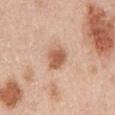| key | value |
|---|---|
| workup | total-body-photography surveillance lesion; no biopsy |
| tile lighting | white-light |
| image | 15 mm crop, total-body photography |
| lesion size | ~3 mm (longest diameter) |
| patient | female, aged 48–52 |
| location | the left upper arm |